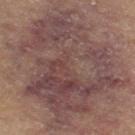• workup · catalogued during a skin exam; not biopsied
• anatomic site · the left thigh
• imaging modality · ~15 mm tile from a whole-body skin photo
• subject · male, approximately 85 years of age or older
• size · about 12 mm